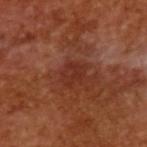Clinical impression:
Captured during whole-body skin photography for melanoma surveillance; the lesion was not biopsied.
Clinical summary:
The patient is a male aged 63–67. This is a cross-polarized tile. A roughly 15 mm field-of-view crop from a total-body skin photograph.